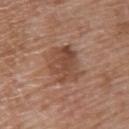Assessment: The lesion was tiled from a total-body skin photograph and was not biopsied. Background: A 15 mm close-up extracted from a 3D total-body photography capture. On the upper back. A female patient roughly 70 years of age.